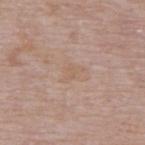Q: Is there a histopathology result?
A: no biopsy performed (imaged during a skin exam)
Q: Patient demographics?
A: male, aged 63–67
Q: What is the anatomic site?
A: the back
Q: What lighting was used for the tile?
A: white-light illumination
Q: What kind of image is this?
A: ~15 mm crop, total-body skin-cancer survey
Q: Lesion size?
A: about 2.5 mm